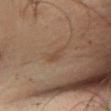Imaged during a routine full-body skin examination; the lesion was not biopsied and no histopathology is available.
A 15 mm crop from a total-body photograph taken for skin-cancer surveillance.
The tile uses cross-polarized illumination.
On the left lower leg.
A male subject in their mid-40s.